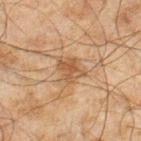Recorded during total-body skin imaging; not selected for excision or biopsy.
The lesion is on the leg.
Measured at roughly 3 mm in maximum diameter.
A male patient in their mid- to late 40s.
A 15 mm close-up tile from a total-body photography series done for melanoma screening.
Captured under cross-polarized illumination.